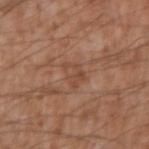No biopsy was performed on this lesion — it was imaged during a full skin examination and was not determined to be concerning. Cropped from a total-body skin-imaging series; the visible field is about 15 mm. Longest diameter approximately 3 mm. Located on the arm. Imaged with white-light lighting. Automated tile analysis of the lesion measured an area of roughly 5 mm², a shape eccentricity near 0.65, and a shape-asymmetry score of about 0.35 (0 = symmetric). The software also gave a border-irregularity index near 3.5/10 and a within-lesion color-variation index near 3.5/10. A male patient, about 75 years old.